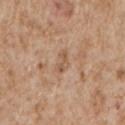| feature | finding |
|---|---|
| notes | total-body-photography surveillance lesion; no biopsy |
| location | the left upper arm |
| subject | male, aged 63–67 |
| acquisition | 15 mm crop, total-body photography |